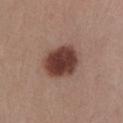| key | value |
|---|---|
| follow-up | total-body-photography surveillance lesion; no biopsy |
| image source | ~15 mm tile from a whole-body skin photo |
| patient | female, in their mid- to late 20s |
| lesion size | ~4.5 mm (longest diameter) |
| location | the chest |
| tile lighting | white-light illumination |